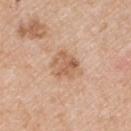biopsy status: total-body-photography surveillance lesion; no biopsy
lesion size: ≈4 mm
acquisition: ~15 mm crop, total-body skin-cancer survey
subject: male, aged 48–52
location: the arm
illumination: white-light illumination